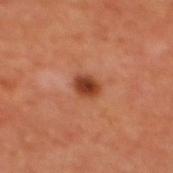patient:
  sex: female
lesion_size:
  long_diameter_mm_approx: 2.5
automated_metrics:
  nevus_likeness_0_100: 95
  lesion_detection_confidence_0_100: 100
image:
  source: total-body photography crop
  field_of_view_mm: 15
site: back